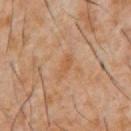patient = male, aged around 60 | anatomic site = the front of the torso | acquisition = 15 mm crop, total-body photography.